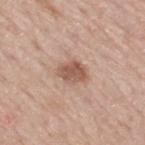{
  "biopsy_status": "not biopsied; imaged during a skin examination",
  "patient": {
    "sex": "male",
    "age_approx": 65
  },
  "site": "mid back",
  "image": {
    "source": "total-body photography crop",
    "field_of_view_mm": 15
  },
  "lighting": "white-light"
}